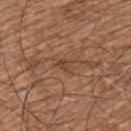The lesion was photographed on a routine skin check and not biopsied; there is no pathology result. A lesion tile, about 15 mm wide, cut from a 3D total-body photograph. On the left upper arm. This is a white-light tile. The patient is a male aged 73–77.